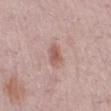This lesion was catalogued during total-body skin photography and was not selected for biopsy.
About 2.5 mm across.
The lesion is on the leg.
The patient is a female in their mid- to late 60s.
Captured under white-light illumination.
The lesion-visualizer software estimated a border-irregularity index near 2/10, a within-lesion color-variation index near 2.5/10, and radial color variation of about 1. It also reported a nevus-likeness score of about 65/100 and a lesion-detection confidence of about 100/100.
A close-up tile cropped from a whole-body skin photograph, about 15 mm across.